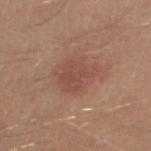Q: What is the imaging modality?
A: ~15 mm crop, total-body skin-cancer survey
Q: Automated lesion metrics?
A: a lesion area of about 12 mm², an outline eccentricity of about 0.55 (0 = round, 1 = elongated), and a symmetry-axis asymmetry near 0.25; a lesion color around L≈49 a*≈22 b*≈27 in CIELAB and a normalized lesion–skin contrast near 5.5; radial color variation of about 0.5
Q: Lesion location?
A: the left lower leg
Q: How was the tile lit?
A: white-light illumination
Q: What are the patient's age and sex?
A: male, aged 28–32